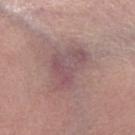workup: imaged on a skin check; not biopsied
patient: female, in their mid- to late 60s
lesion diameter: ~5.5 mm (longest diameter)
TBP lesion metrics: a mean CIELAB color near L≈52 a*≈20 b*≈17, about 7 CIELAB-L* units darker than the surrounding skin, and a lesion-to-skin contrast of about 6 (normalized; higher = more distinct); a within-lesion color-variation index near 5.5/10 and peripheral color asymmetry of about 2; an automated nevus-likeness rating near 0 out of 100 and lesion-presence confidence of about 80/100
location: the left forearm
tile lighting: white-light
image: ~15 mm crop, total-body skin-cancer survey